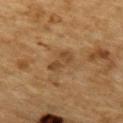Q: Was this lesion biopsied?
A: imaged on a skin check; not biopsied
Q: Automated lesion metrics?
A: an area of roughly 5 mm² and a shape eccentricity near 0.85; a lesion color around L≈38 a*≈16 b*≈32 in CIELAB, roughly 7 lightness units darker than nearby skin, and a normalized lesion–skin contrast near 6.5; a nevus-likeness score of about 5/100 and a lesion-detection confidence of about 100/100
Q: What is the anatomic site?
A: the mid back
Q: What kind of image is this?
A: total-body-photography crop, ~15 mm field of view
Q: What are the patient's age and sex?
A: male, about 85 years old or older
Q: What is the lesion's diameter?
A: about 3 mm
Q: What lighting was used for the tile?
A: cross-polarized